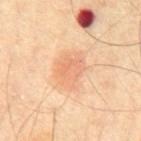Impression:
Part of a total-body skin-imaging series; this lesion was reviewed on a skin check and was not flagged for biopsy.
Background:
The lesion is on the abdomen. A male patient, in their mid- to late 60s. Approximately 4.5 mm at its widest. Cropped from a total-body skin-imaging series; the visible field is about 15 mm.